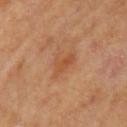Q: Was this lesion biopsied?
A: no biopsy performed (imaged during a skin exam)
Q: What lighting was used for the tile?
A: cross-polarized illumination
Q: Automated lesion metrics?
A: an eccentricity of roughly 0.8; a within-lesion color-variation index near 2.5/10 and radial color variation of about 0.5; a classifier nevus-likeness of about 0/100 and a lesion-detection confidence of about 100/100
Q: Who is the patient?
A: female, roughly 65 years of age
Q: Where on the body is the lesion?
A: the left upper arm
Q: How large is the lesion?
A: ~3 mm (longest diameter)
Q: What kind of image is this?
A: total-body-photography crop, ~15 mm field of view